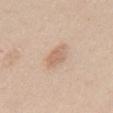Impression: The lesion was tiled from a total-body skin photograph and was not biopsied. Context: Cropped from a whole-body photographic skin survey; the tile spans about 15 mm. This is a white-light tile. The lesion's longest dimension is about 3.5 mm. The lesion is located on the front of the torso. A male patient, aged 33 to 37.